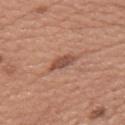Impression: No biopsy was performed on this lesion — it was imaged during a full skin examination and was not determined to be concerning. Context: The subject is a female in their 60s. Approximately 3.5 mm at its widest. This is a white-light tile. A close-up tile cropped from a whole-body skin photograph, about 15 mm across. The lesion-visualizer software estimated an area of roughly 4.5 mm², an eccentricity of roughly 0.85, and a shape-asymmetry score of about 0.25 (0 = symmetric). The analysis additionally found a border-irregularity index near 3/10, a within-lesion color-variation index near 1.5/10, and radial color variation of about 0.5. And it measured a classifier nevus-likeness of about 45/100 and a detector confidence of about 100 out of 100 that the crop contains a lesion. The lesion is on the right upper arm.